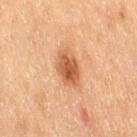The lesion was photographed on a routine skin check and not biopsied; there is no pathology result.
The lesion is located on the right thigh.
A region of skin cropped from a whole-body photographic capture, roughly 15 mm wide.
The patient is a female about 55 years old.